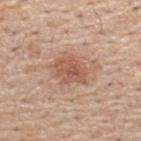Findings:
• biopsy status · catalogued during a skin exam; not biopsied
• TBP lesion metrics · border irregularity of about 2.5 on a 0–10 scale, a within-lesion color-variation index near 3.5/10, and a peripheral color-asymmetry measure near 1
• image · 15 mm crop, total-body photography
• lighting · white-light illumination
• lesion diameter · ~4 mm (longest diameter)
• patient · male, approximately 55 years of age
• site · the upper back